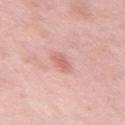The lesion was photographed on a routine skin check and not biopsied; there is no pathology result. The recorded lesion diameter is about 2.5 mm. The patient is a male roughly 55 years of age. Captured under white-light illumination. Automated image analysis of the tile measured a footprint of about 3 mm², an outline eccentricity of about 0.85 (0 = round, 1 = elongated), and two-axis asymmetry of about 0.3. And it measured a lesion color around L≈64 a*≈25 b*≈26 in CIELAB, a lesion–skin lightness drop of about 9, and a normalized lesion–skin contrast near 6. A region of skin cropped from a whole-body photographic capture, roughly 15 mm wide. The lesion is located on the mid back.